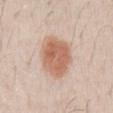<lesion>
  <biopsy_status>not biopsied; imaged during a skin examination</biopsy_status>
  <patient>
    <sex>male</sex>
    <age_approx>30</age_approx>
  </patient>
  <image>
    <source>total-body photography crop</source>
    <field_of_view_mm>15</field_of_view_mm>
  </image>
  <lighting>white-light</lighting>
  <lesion_size>
    <long_diameter_mm_approx>6.0</long_diameter_mm_approx>
  </lesion_size>
  <site>chest</site>
</lesion>